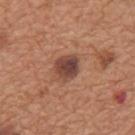image source: 15 mm crop, total-body photography; subject: male, approximately 65 years of age; body site: the mid back.